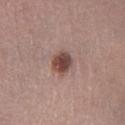Clinical impression:
No biopsy was performed on this lesion — it was imaged during a full skin examination and was not determined to be concerning.
Background:
The recorded lesion diameter is about 3 mm. Cropped from a total-body skin-imaging series; the visible field is about 15 mm. On the left lower leg. A female patient aged around 65. This is a white-light tile.